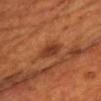On the chest. A 15 mm crop from a total-body photograph taken for skin-cancer surveillance. A male patient, aged approximately 55. This is a cross-polarized tile.